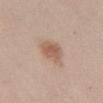Captured during whole-body skin photography for melanoma surveillance; the lesion was not biopsied. A roughly 15 mm field-of-view crop from a total-body skin photograph. A female subject in their 40s. The lesion-visualizer software estimated a lesion area of about 6.5 mm². The software also gave a border-irregularity rating of about 2.5/10 and a color-variation rating of about 2.5/10. Imaged with white-light lighting. About 3 mm across. The lesion is on the abdomen.